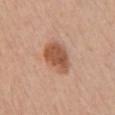biopsy_status: not biopsied; imaged during a skin examination
site: chest
automated_metrics:
  cielab_L: 54
  cielab_a: 23
  cielab_b: 32
  vs_skin_darker_L: 13.0
  border_irregularity_0_10: 2.0
  color_variation_0_10: 3.5
image:
  source: total-body photography crop
  field_of_view_mm: 15
patient:
  sex: male
  age_approx: 40
lighting: white-light